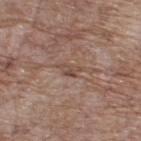| key | value |
|---|---|
| workup | total-body-photography surveillance lesion; no biopsy |
| lesion size | ≈2.5 mm |
| location | the back |
| patient | male, in their 70s |
| image source | 15 mm crop, total-body photography |
| tile lighting | white-light |